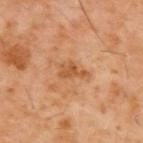| feature | finding |
|---|---|
| follow-up | imaged on a skin check; not biopsied |
| image-analysis metrics | a shape eccentricity near 0.85; an average lesion color of about L≈51 a*≈23 b*≈38 (CIELAB) and about 9 CIELAB-L* units darker than the surrounding skin |
| body site | the upper back |
| patient | male, in their 60s |
| tile lighting | cross-polarized illumination |
| image | total-body-photography crop, ~15 mm field of view |
| lesion size | ~3.5 mm (longest diameter) |